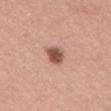{"biopsy_status": "not biopsied; imaged during a skin examination", "site": "abdomen", "patient": {"sex": "male", "age_approx": 35}, "image": {"source": "total-body photography crop", "field_of_view_mm": 15}}